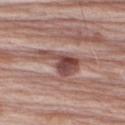Recorded during total-body skin imaging; not selected for excision or biopsy. An algorithmic analysis of the crop reported border irregularity of about 7 on a 0–10 scale, a color-variation rating of about 5.5/10, and peripheral color asymmetry of about 2. This is a white-light tile. Located on the right upper arm. Cropped from a whole-body photographic skin survey; the tile spans about 15 mm. A male patient, approximately 65 years of age.